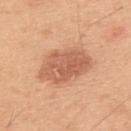Case summary:
* biopsy status · no biopsy performed (imaged during a skin exam)
* body site · the upper back
* image source · ~15 mm crop, total-body skin-cancer survey
* patient · male, aged around 50